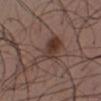– workup: total-body-photography surveillance lesion; no biopsy
– lesion diameter: ≈6 mm
– anatomic site: the chest
– imaging modality: total-body-photography crop, ~15 mm field of view
– subject: male, aged approximately 40